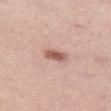Q: Was this lesion biopsied?
A: no biopsy performed (imaged during a skin exam)
Q: How was this image acquired?
A: ~15 mm crop, total-body skin-cancer survey
Q: What did automated image analysis measure?
A: a border-irregularity rating of about 2/10, internal color variation of about 2.5 on a 0–10 scale, and peripheral color asymmetry of about 1; an automated nevus-likeness rating near 90 out of 100 and a lesion-detection confidence of about 100/100
Q: How large is the lesion?
A: about 2.5 mm
Q: Illumination type?
A: white-light illumination
Q: What are the patient's age and sex?
A: female, aged 28 to 32
Q: What is the anatomic site?
A: the left thigh